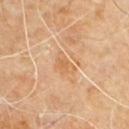follow-up: no biopsy performed (imaged during a skin exam)
site: the upper back
acquisition: 15 mm crop, total-body photography
illumination: cross-polarized
subject: male, aged approximately 60
lesion size: ~3 mm (longest diameter)
TBP lesion metrics: a shape eccentricity near 0.75; a nevus-likeness score of about 0/100 and a lesion-detection confidence of about 100/100